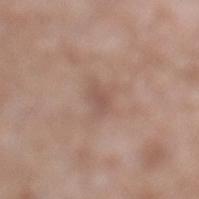Part of a total-body skin-imaging series; this lesion was reviewed on a skin check and was not flagged for biopsy. About 3 mm across. The patient is a male in their 60s. This image is a 15 mm lesion crop taken from a total-body photograph. From the leg. The tile uses white-light illumination.